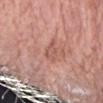Recorded during total-body skin imaging; not selected for excision or biopsy. The patient is a female aged 68–72. Captured under white-light illumination. The lesion is located on the arm. The lesion's longest dimension is about 3 mm. A 15 mm close-up tile from a total-body photography series done for melanoma screening.